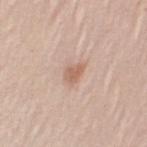{"image": {"source": "total-body photography crop", "field_of_view_mm": 15}, "site": "left upper arm", "automated_metrics": {"area_mm2_approx": 3.5, "eccentricity": 0.8, "shape_asymmetry": 0.3}, "lesion_size": {"long_diameter_mm_approx": 2.5}, "lighting": "white-light", "patient": {"sex": "female", "age_approx": 30}}